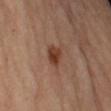follow-up = catalogued during a skin exam; not biopsied
size = ~3 mm (longest diameter)
tile lighting = cross-polarized illumination
location = the arm
subject = female
acquisition = 15 mm crop, total-body photography